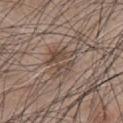biopsy status — imaged on a skin check; not biopsied | image-analysis metrics — a lesion area of about 9.5 mm², a shape eccentricity near 0.4, and a symmetry-axis asymmetry near 0.4; an average lesion color of about L≈47 a*≈14 b*≈23 (CIELAB) and a normalized lesion–skin contrast near 6.5; border irregularity of about 5.5 on a 0–10 scale, a color-variation rating of about 5/10, and peripheral color asymmetry of about 2 | size — about 4 mm | patient — male, aged approximately 75 | lighting — white-light illumination | anatomic site — the abdomen | acquisition — 15 mm crop, total-body photography.